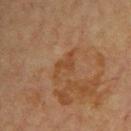workup: catalogued during a skin exam; not biopsied | anatomic site: the upper back | patient: male, approximately 60 years of age | acquisition: ~15 mm crop, total-body skin-cancer survey.